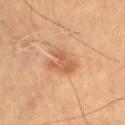Captured under cross-polarized illumination. A roughly 15 mm field-of-view crop from a total-body skin photograph. Approximately 4 mm at its widest. A male patient, roughly 55 years of age. Located on the chest.